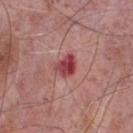Clinical impression:
The lesion was photographed on a routine skin check and not biopsied; there is no pathology result.
Clinical summary:
Measured at roughly 3 mm in maximum diameter. A roughly 15 mm field-of-view crop from a total-body skin photograph. Located on the front of the torso. A male subject, aged approximately 75. Captured under white-light illumination.